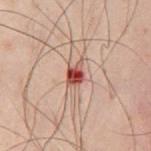Q: Was this lesion biopsied?
A: catalogued during a skin exam; not biopsied
Q: Patient demographics?
A: male, about 50 years old
Q: How was this image acquired?
A: ~15 mm tile from a whole-body skin photo
Q: What is the anatomic site?
A: the front of the torso
Q: What did automated image analysis measure?
A: a lesion area of about 4 mm² and two-axis asymmetry of about 0.2